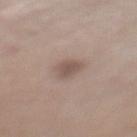Q: Was a biopsy performed?
A: no biopsy performed (imaged during a skin exam)
Q: What kind of image is this?
A: total-body-photography crop, ~15 mm field of view
Q: Lesion size?
A: ~3 mm (longest diameter)
Q: Illumination type?
A: white-light illumination
Q: What is the anatomic site?
A: the left lower leg
Q: Who is the patient?
A: female, aged around 65